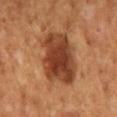{"biopsy_status": "not biopsied; imaged during a skin examination", "lighting": "cross-polarized", "lesion_size": {"long_diameter_mm_approx": 6.5}, "automated_metrics": {"area_mm2_approx": 25.0, "eccentricity": 0.75, "shape_asymmetry": 0.2, "border_irregularity_0_10": 2.5, "peripheral_color_asymmetry": 1.5, "nevus_likeness_0_100": 95, "lesion_detection_confidence_0_100": 100}, "image": {"source": "total-body photography crop", "field_of_view_mm": 15}, "patient": {"sex": "male", "age_approx": 60}, "site": "abdomen"}